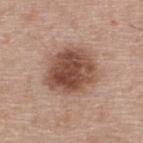Q: Was this lesion biopsied?
A: imaged on a skin check; not biopsied
Q: Patient demographics?
A: male, aged 53–57
Q: What is the imaging modality?
A: 15 mm crop, total-body photography